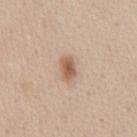Impression:
Part of a total-body skin-imaging series; this lesion was reviewed on a skin check and was not flagged for biopsy.